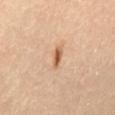Notes:
- workup: no biopsy performed (imaged during a skin exam)
- subject: female, aged 63–67
- acquisition: ~15 mm tile from a whole-body skin photo
- body site: the mid back